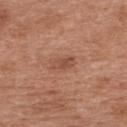<case>
<biopsy_status>not biopsied; imaged during a skin examination</biopsy_status>
<lighting>white-light</lighting>
<site>upper back</site>
<patient>
  <sex>male</sex>
  <age_approx>30</age_approx>
</patient>
<lesion_size>
  <long_diameter_mm_approx>2.5</long_diameter_mm_approx>
</lesion_size>
<image>
  <source>total-body photography crop</source>
  <field_of_view_mm>15</field_of_view_mm>
</image>
</case>